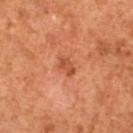Q: Was a biopsy performed?
A: total-body-photography surveillance lesion; no biopsy
Q: Lesion location?
A: the left lower leg
Q: Who is the patient?
A: female, in their mid- to late 60s
Q: How was this image acquired?
A: ~15 mm crop, total-body skin-cancer survey